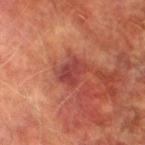Impression:
The lesion was tiled from a total-body skin photograph and was not biopsied.
Background:
From the left thigh. Cropped from a whole-body photographic skin survey; the tile spans about 15 mm. A male patient aged 73 to 77.